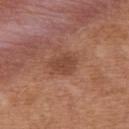Assessment: Imaged during a routine full-body skin examination; the lesion was not biopsied and no histopathology is available. Acquisition and patient details: The total-body-photography lesion software estimated a footprint of about 5 mm² and a shape-asymmetry score of about 0.3 (0 = symmetric). It also reported an automated nevus-likeness rating near 40 out of 100 and a detector confidence of about 100 out of 100 that the crop contains a lesion. A roughly 15 mm field-of-view crop from a total-body skin photograph. The subject is a female aged approximately 40. From the upper back. The recorded lesion diameter is about 3 mm. This is a white-light tile.